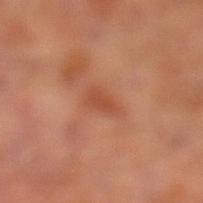Case summary:
* notes · catalogued during a skin exam; not biopsied
* subject · male, roughly 65 years of age
* image source · ~15 mm crop, total-body skin-cancer survey
* anatomic site · the left lower leg
* diameter · ~3 mm (longest diameter)